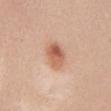{
  "biopsy_status": "not biopsied; imaged during a skin examination",
  "lesion_size": {
    "long_diameter_mm_approx": 4.0
  },
  "automated_metrics": {
    "area_mm2_approx": 7.5,
    "eccentricity": 0.8,
    "cielab_L": 61,
    "cielab_a": 23,
    "cielab_b": 32,
    "vs_skin_darker_L": 12.0,
    "vs_skin_contrast_norm": 8.0,
    "border_irregularity_0_10": 1.5,
    "color_variation_0_10": 7.5,
    "peripheral_color_asymmetry": 3.0
  },
  "image": {
    "source": "total-body photography crop",
    "field_of_view_mm": 15
  },
  "patient": {
    "sex": "female",
    "age_approx": 35
  },
  "lighting": "white-light",
  "site": "chest"
}